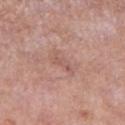A female patient, aged around 70. This image is a 15 mm lesion crop taken from a total-body photograph. On the right lower leg.